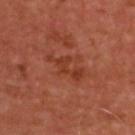Case summary:
• notes — total-body-photography surveillance lesion; no biopsy
• lesion diameter — ~4.5 mm (longest diameter)
• automated lesion analysis — about 7 CIELAB-L* units darker than the surrounding skin and a normalized border contrast of about 6.5; a border-irregularity rating of about 8.5/10 and a peripheral color-asymmetry measure near 0; lesion-presence confidence of about 100/100
• image — ~15 mm crop, total-body skin-cancer survey
• location — the upper back
• patient — male, aged around 50